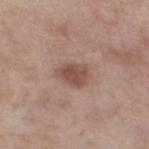Q: Was this lesion biopsied?
A: imaged on a skin check; not biopsied
Q: Lesion size?
A: ≈3.5 mm
Q: Where on the body is the lesion?
A: the left thigh
Q: What is the imaging modality?
A: 15 mm crop, total-body photography
Q: Patient demographics?
A: female, approximately 55 years of age
Q: What lighting was used for the tile?
A: white-light